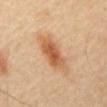{"biopsy_status": "not biopsied; imaged during a skin examination", "site": "abdomen", "automated_metrics": {"eccentricity": 0.9, "shape_asymmetry": 0.25, "cielab_L": 55, "cielab_a": 22, "cielab_b": 36, "vs_skin_darker_L": 12.0, "vs_skin_contrast_norm": 8.0}, "image": {"source": "total-body photography crop", "field_of_view_mm": 15}, "patient": {"sex": "male", "age_approx": 45}, "lesion_size": {"long_diameter_mm_approx": 6.0}}